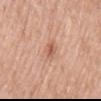The lesion was tiled from a total-body skin photograph and was not biopsied.
A female subject, roughly 65 years of age.
The lesion-visualizer software estimated a classifier nevus-likeness of about 55/100 and lesion-presence confidence of about 100/100.
Located on the chest.
Captured under white-light illumination.
A 15 mm close-up extracted from a 3D total-body photography capture.
The recorded lesion diameter is about 2.5 mm.